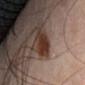This lesion was catalogued during total-body skin photography and was not selected for biopsy.
Imaged with cross-polarized lighting.
Cropped from a total-body skin-imaging series; the visible field is about 15 mm.
Located on the front of the torso.
The patient is a male roughly 65 years of age.
Measured at roughly 4.5 mm in maximum diameter.